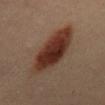Q: Is there a histopathology result?
A: no biopsy performed (imaged during a skin exam)